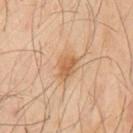biopsy_status: not biopsied; imaged during a skin examination
automated_metrics:
  nevus_likeness_0_100: 70
  lesion_detection_confidence_0_100: 100
patient:
  sex: male
  age_approx: 60
lighting: cross-polarized
lesion_size:
  long_diameter_mm_approx: 3.0
site: front of the torso
image:
  source: total-body photography crop
  field_of_view_mm: 15